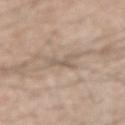Impression: This lesion was catalogued during total-body skin photography and was not selected for biopsy. Acquisition and patient details: Measured at roughly 3.5 mm in maximum diameter. A male patient aged 63 to 67. Captured under white-light illumination. From the right upper arm. A 15 mm close-up tile from a total-body photography series done for melanoma screening.